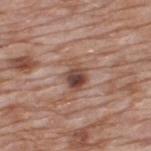A 15 mm crop from a total-body photograph taken for skin-cancer surveillance. Measured at roughly 3.5 mm in maximum diameter. Located on the upper back. The subject is a male about 60 years old. An algorithmic analysis of the crop reported a footprint of about 6.5 mm², an outline eccentricity of about 0.65 (0 = round, 1 = elongated), and a symmetry-axis asymmetry near 0.35. The software also gave a border-irregularity rating of about 3.5/10, a within-lesion color-variation index near 8.5/10, and a peripheral color-asymmetry measure near 3.